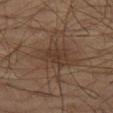Part of a total-body skin-imaging series; this lesion was reviewed on a skin check and was not flagged for biopsy.
A 15 mm close-up tile from a total-body photography series done for melanoma screening.
A male subject, roughly 55 years of age.
Automated image analysis of the tile measured a border-irregularity index near 3.5/10 and a color-variation rating of about 2/10. It also reported an automated nevus-likeness rating near 0 out of 100.
This is a cross-polarized tile.
The lesion is located on the left thigh.
Longest diameter approximately 3.5 mm.